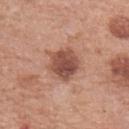biopsy status: imaged on a skin check; not biopsied
lighting: white-light illumination
imaging modality: 15 mm crop, total-body photography
subject: female, about 60 years old
TBP lesion metrics: a border-irregularity index near 2/10, internal color variation of about 4 on a 0–10 scale, and peripheral color asymmetry of about 1.5
body site: the left forearm
diameter: about 4 mm